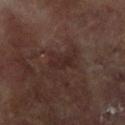Captured during whole-body skin photography for melanoma surveillance; the lesion was not biopsied. The lesion is on the left arm. A close-up tile cropped from a whole-body skin photograph, about 15 mm across. The recorded lesion diameter is about 3 mm. The subject is a female about 80 years old.